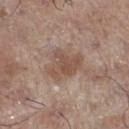Assessment: No biopsy was performed on this lesion — it was imaged during a full skin examination and was not determined to be concerning. Background: A male subject, aged approximately 70. The tile uses white-light illumination. A region of skin cropped from a whole-body photographic capture, roughly 15 mm wide. The lesion is on the left lower leg. Longest diameter approximately 5 mm.